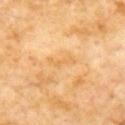Captured during whole-body skin photography for melanoma surveillance; the lesion was not biopsied. The lesion is located on the chest. The tile uses cross-polarized illumination. The patient is a male in their 60s. Approximately 3 mm at its widest. A roughly 15 mm field-of-view crop from a total-body skin photograph.